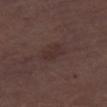Part of a total-body skin-imaging series; this lesion was reviewed on a skin check and was not flagged for biopsy. Located on the right thigh. The patient is a male in their 70s. The total-body-photography lesion software estimated a lesion area of about 5 mm², an outline eccentricity of about 0.85 (0 = round, 1 = elongated), and a symmetry-axis asymmetry near 0.25. It also reported an average lesion color of about L≈30 a*≈16 b*≈17 (CIELAB), about 5 CIELAB-L* units darker than the surrounding skin, and a normalized border contrast of about 5.5. It also reported a border-irregularity rating of about 3/10, a within-lesion color-variation index near 1.5/10, and peripheral color asymmetry of about 0.5. Measured at roughly 3.5 mm in maximum diameter. A region of skin cropped from a whole-body photographic capture, roughly 15 mm wide. The tile uses white-light illumination.